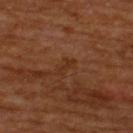biopsy_status: not biopsied; imaged during a skin examination
automated_metrics:
  area_mm2_approx: 1.5
  eccentricity: 0.95
  shape_asymmetry: 0.5
  nevus_likeness_0_100: 0
  lesion_detection_confidence_0_100: 100
site: upper back
lesion_size:
  long_diameter_mm_approx: 2.5
patient:
  sex: male
  age_approx: 65
image:
  source: total-body photography crop
  field_of_view_mm: 15
lighting: cross-polarized